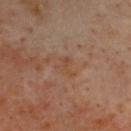{
  "biopsy_status": "not biopsied; imaged during a skin examination",
  "site": "head or neck",
  "automated_metrics": {
    "border_irregularity_0_10": 7.0,
    "color_variation_0_10": 0.0
  },
  "image": {
    "source": "total-body photography crop",
    "field_of_view_mm": 15
  },
  "lighting": "cross-polarized",
  "patient": {
    "sex": "male",
    "age_approx": 65
  }
}